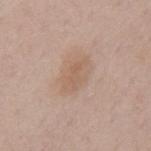<tbp_lesion>
<biopsy_status>not biopsied; imaged during a skin examination</biopsy_status>
<site>back</site>
<image>
  <source>total-body photography crop</source>
  <field_of_view_mm>15</field_of_view_mm>
</image>
<patient>
  <sex>male</sex>
  <age_approx>70</age_approx>
</patient>
</tbp_lesion>